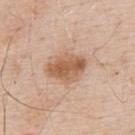Case summary:
– workup: no biopsy performed (imaged during a skin exam)
– patient: male, aged approximately 60
– location: the back
– lesion size: ~4.5 mm (longest diameter)
– tile lighting: white-light illumination
– imaging modality: 15 mm crop, total-body photography